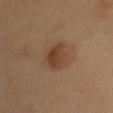Imaged during a routine full-body skin examination; the lesion was not biopsied and no histopathology is available.